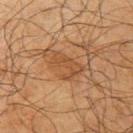follow-up: no biopsy performed (imaged during a skin exam) | patient: male, aged approximately 65 | site: the left upper arm | image: 15 mm crop, total-body photography | lesion diameter: about 4 mm | tile lighting: cross-polarized illumination.